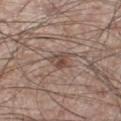| field | value |
|---|---|
| biopsy status | total-body-photography surveillance lesion; no biopsy |
| subject | male, aged 58 to 62 |
| acquisition | ~15 mm crop, total-body skin-cancer survey |
| lesion size | ≈2.5 mm |
| tile lighting | white-light illumination |
| site | the left thigh |
| image-analysis metrics | a footprint of about 4 mm² and a shape-asymmetry score of about 0.4 (0 = symmetric); a lesion color around L≈47 a*≈16 b*≈23 in CIELAB, roughly 10 lightness units darker than nearby skin, and a normalized lesion–skin contrast near 7.5; a border-irregularity rating of about 4.5/10, internal color variation of about 5.5 on a 0–10 scale, and a peripheral color-asymmetry measure near 2 |